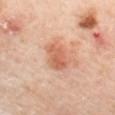Captured during whole-body skin photography for melanoma surveillance; the lesion was not biopsied. A female subject, in their 60s. A lesion tile, about 15 mm wide, cut from a 3D total-body photograph. About 3.5 mm across. Captured under cross-polarized illumination. From the mid back.